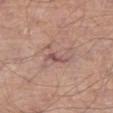The lesion was tiled from a total-body skin photograph and was not biopsied. The tile uses white-light illumination. A male subject, approximately 80 years of age. A roughly 15 mm field-of-view crop from a total-body skin photograph. About 4 mm across. The lesion is located on the right thigh.